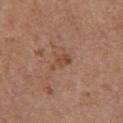Context:
The recorded lesion diameter is about 3 mm. An algorithmic analysis of the crop reported a lesion area of about 3.5 mm², an eccentricity of roughly 0.85, and a shape-asymmetry score of about 0.4 (0 = symmetric). It also reported a mean CIELAB color near L≈47 a*≈21 b*≈30, roughly 7 lightness units darker than nearby skin, and a normalized lesion–skin contrast near 6. The software also gave a border-irregularity rating of about 4.5/10 and peripheral color asymmetry of about 0.5. A lesion tile, about 15 mm wide, cut from a 3D total-body photograph. A female patient, aged approximately 65. The tile uses white-light illumination. On the chest.